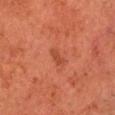Clinical impression: No biopsy was performed on this lesion — it was imaged during a full skin examination and was not determined to be concerning. Context: The tile uses cross-polarized illumination. The lesion-visualizer software estimated a lesion area of about 2.5 mm² and a shape eccentricity near 0.9. And it measured an average lesion color of about L≈37 a*≈26 b*≈30 (CIELAB), roughly 6 lightness units darker than nearby skin, and a normalized lesion–skin contrast near 5.5. It also reported a border-irregularity rating of about 4/10, internal color variation of about 0 on a 0–10 scale, and a peripheral color-asymmetry measure near 0. The subject is a male roughly 50 years of age. Measured at roughly 3 mm in maximum diameter. The lesion is on the head or neck. A lesion tile, about 15 mm wide, cut from a 3D total-body photograph.